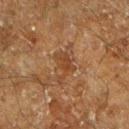biopsy status = total-body-photography surveillance lesion; no biopsy